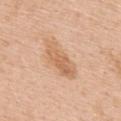No biopsy was performed on this lesion — it was imaged during a full skin examination and was not determined to be concerning. Cropped from a whole-body photographic skin survey; the tile spans about 15 mm. This is a white-light tile. Located on the upper back. An algorithmic analysis of the crop reported border irregularity of about 4 on a 0–10 scale, internal color variation of about 3.5 on a 0–10 scale, and radial color variation of about 1.5. The analysis additionally found lesion-presence confidence of about 100/100. A female subject approximately 65 years of age. Longest diameter approximately 6 mm.